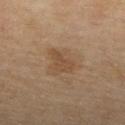Imaged during a routine full-body skin examination; the lesion was not biopsied and no histopathology is available.
A female patient, aged around 55.
From the right lower leg.
Longest diameter approximately 3.5 mm.
The total-body-photography lesion software estimated roughly 6 lightness units darker than nearby skin and a normalized border contrast of about 5.5. And it measured a border-irregularity rating of about 3.5/10 and a within-lesion color-variation index near 1/10. The software also gave an automated nevus-likeness rating near 10 out of 100.
The tile uses cross-polarized illumination.
This image is a 15 mm lesion crop taken from a total-body photograph.